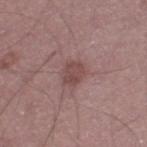<record>
  <biopsy_status>not biopsied; imaged during a skin examination</biopsy_status>
  <image>
    <source>total-body photography crop</source>
    <field_of_view_mm>15</field_of_view_mm>
  </image>
  <automated_metrics>
    <area_mm2_approx>5.0</area_mm2_approx>
    <eccentricity>0.55</eccentricity>
    <shape_asymmetry>0.25</shape_asymmetry>
    <cielab_L>46</cielab_L>
    <cielab_a>20</cielab_a>
    <cielab_b>20</cielab_b>
    <vs_skin_darker_L>9.0</vs_skin_darker_L>
  </automated_metrics>
  <patient>
    <sex>male</sex>
    <age_approx>60</age_approx>
  </patient>
  <site>left thigh</site>
  <lesion_size>
    <long_diameter_mm_approx>2.5</long_diameter_mm_approx>
  </lesion_size>
</record>